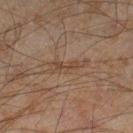Impression: Part of a total-body skin-imaging series; this lesion was reviewed on a skin check and was not flagged for biopsy. Image and clinical context: A region of skin cropped from a whole-body photographic capture, roughly 15 mm wide. On the right lower leg. Approximately 4.5 mm at its widest. Automated tile analysis of the lesion measured roughly 5 lightness units darker than nearby skin and a lesion-to-skin contrast of about 5.5 (normalized; higher = more distinct). The analysis additionally found a border-irregularity index near 6/10 and a peripheral color-asymmetry measure near 0. And it measured a nevus-likeness score of about 0/100. The subject is a male about 45 years old. Captured under cross-polarized illumination.